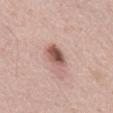Recorded during total-body skin imaging; not selected for excision or biopsy. Located on the mid back. This is a white-light tile. A 15 mm close-up tile from a total-body photography series done for melanoma screening. A male subject, about 65 years old. Longest diameter approximately 3 mm.